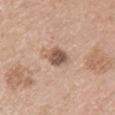biopsy_status: not biopsied; imaged during a skin examination
image:
  source: total-body photography crop
  field_of_view_mm: 15
automated_metrics:
  eccentricity: 0.55
  shape_asymmetry: 0.15
  cielab_L: 54
  cielab_a: 19
  cielab_b: 27
  vs_skin_darker_L: 14.0
  vs_skin_contrast_norm: 9.0
  nevus_likeness_0_100: 65
  lesion_detection_confidence_0_100: 100
patient:
  sex: female
  age_approx: 55
lighting: white-light
site: arm
lesion_size:
  long_diameter_mm_approx: 3.0